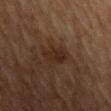This lesion was catalogued during total-body skin photography and was not selected for biopsy. Longest diameter approximately 3 mm. Imaged with cross-polarized lighting. A male patient, approximately 85 years of age or older. A lesion tile, about 15 mm wide, cut from a 3D total-body photograph. The lesion is located on the back. The lesion-visualizer software estimated a lesion area of about 5.5 mm², an eccentricity of roughly 0.65, and a shape-asymmetry score of about 0.25 (0 = symmetric). And it measured an average lesion color of about L≈22 a*≈15 b*≈22 (CIELAB) and roughly 5 lightness units darker than nearby skin. And it measured border irregularity of about 2.5 on a 0–10 scale and a within-lesion color-variation index near 2.5/10.